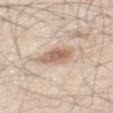Clinical summary: A 15 mm close-up tile from a total-body photography series done for melanoma screening. An algorithmic analysis of the crop reported a lesion area of about 8.5 mm², a shape eccentricity near 0.8, and a symmetry-axis asymmetry near 0.25. And it measured a border-irregularity rating of about 2.5/10 and peripheral color asymmetry of about 1. A male patient about 60 years old. The recorded lesion diameter is about 4.5 mm. The lesion is on the mid back.